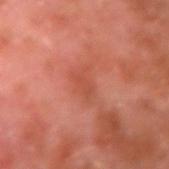Q: Is there a histopathology result?
A: no biopsy performed (imaged during a skin exam)